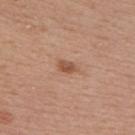Findings:
* notes — catalogued during a skin exam; not biopsied
* location — the right upper arm
* lesion size — ~2.5 mm (longest diameter)
* image source — total-body-photography crop, ~15 mm field of view
* patient — female, aged 38 to 42
* TBP lesion metrics — peripheral color asymmetry of about 0.5; a nevus-likeness score of about 85/100 and a detector confidence of about 100 out of 100 that the crop contains a lesion
* lighting — white-light illumination